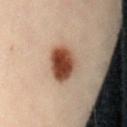The lesion was tiled from a total-body skin photograph and was not biopsied. Measured at roughly 4.5 mm in maximum diameter. Captured under cross-polarized illumination. A female subject aged 28 to 32. The lesion is on the lower back. The lesion-visualizer software estimated an outline eccentricity of about 0.65 (0 = round, 1 = elongated) and a shape-asymmetry score of about 0.2 (0 = symmetric). And it measured a lesion color around L≈37 a*≈19 b*≈25 in CIELAB, about 16 CIELAB-L* units darker than the surrounding skin, and a normalized border contrast of about 13.5. The software also gave a border-irregularity index near 2/10, a within-lesion color-variation index near 6/10, and a peripheral color-asymmetry measure near 2. The software also gave a nevus-likeness score of about 100/100. A roughly 15 mm field-of-view crop from a total-body skin photograph.